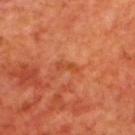Assessment:
Captured during whole-body skin photography for melanoma surveillance; the lesion was not biopsied.
Context:
Captured under cross-polarized illumination. A 15 mm close-up tile from a total-body photography series done for melanoma screening. Located on the upper back. A male patient, aged 68–72.